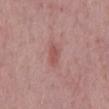Impression:
The lesion was tiled from a total-body skin photograph and was not biopsied.
Clinical summary:
Cropped from a total-body skin-imaging series; the visible field is about 15 mm. Located on the mid back. Captured under white-light illumination. Longest diameter approximately 3.5 mm. The subject is a male approximately 60 years of age.